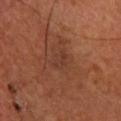<lesion>
  <biopsy_status>not biopsied; imaged during a skin examination</biopsy_status>
  <automated_metrics>
    <nevus_likeness_0_100>0</nevus_likeness_0_100>
  </automated_metrics>
  <site>right lower leg</site>
  <lighting>cross-polarized</lighting>
  <patient>
    <sex>male</sex>
    <age_approx>55</age_approx>
  </patient>
  <image>
    <source>total-body photography crop</source>
    <field_of_view_mm>15</field_of_view_mm>
  </image>
</lesion>